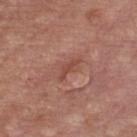Part of a total-body skin-imaging series; this lesion was reviewed on a skin check and was not flagged for biopsy. The lesion is on the chest. A male subject about 55 years old. Cropped from a whole-body photographic skin survey; the tile spans about 15 mm. The tile uses white-light illumination.